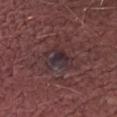Imaged during a routine full-body skin examination; the lesion was not biopsied and no histopathology is available. This is a white-light tile. A male patient in their 50s. On the right thigh. Longest diameter approximately 4 mm. A region of skin cropped from a whole-body photographic capture, roughly 15 mm wide.